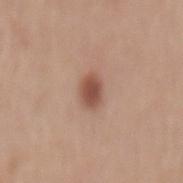Clinical summary: The lesion is located on the mid back. A close-up tile cropped from a whole-body skin photograph, about 15 mm across. Automated image analysis of the tile measured an area of roughly 5 mm² and a shape-asymmetry score of about 0.2 (0 = symmetric). The analysis additionally found a lesion color around L≈51 a*≈21 b*≈27 in CIELAB, roughly 13 lightness units darker than nearby skin, and a normalized lesion–skin contrast near 9. The analysis additionally found internal color variation of about 2.5 on a 0–10 scale and peripheral color asymmetry of about 0.5. The analysis additionally found a lesion-detection confidence of about 100/100. The tile uses white-light illumination. Measured at roughly 3 mm in maximum diameter. A male subject aged 63 to 67.